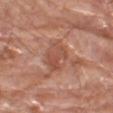<tbp_lesion>
  <biopsy_status>not biopsied; imaged during a skin examination</biopsy_status>
  <patient>
    <sex>male</sex>
    <age_approx>80</age_approx>
  </patient>
  <automated_metrics>
    <color_variation_0_10>5.0</color_variation_0_10>
    <peripheral_color_asymmetry>2.0</peripheral_color_asymmetry>
    <nevus_likeness_0_100>5</nevus_likeness_0_100>
  </automated_metrics>
  <site>left thigh</site>
  <image>
    <source>total-body photography crop</source>
    <field_of_view_mm>15</field_of_view_mm>
  </image>
  <lighting>white-light</lighting>
</tbp_lesion>